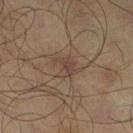Q: Was a biopsy performed?
A: total-body-photography surveillance lesion; no biopsy
Q: What is the imaging modality?
A: 15 mm crop, total-body photography
Q: What are the patient's age and sex?
A: male, in their mid- to late 60s
Q: Lesion size?
A: about 2.5 mm
Q: What is the anatomic site?
A: the right lower leg
Q: Illumination type?
A: cross-polarized